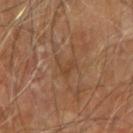patient: male, roughly 70 years of age
image source: ~15 mm tile from a whole-body skin photo
illumination: cross-polarized
anatomic site: the arm
TBP lesion metrics: a mean CIELAB color near L≈42 a*≈19 b*≈31, a lesion–skin lightness drop of about 5, and a normalized border contrast of about 4.5; border irregularity of about 7.5 on a 0–10 scale, internal color variation of about 2 on a 0–10 scale, and peripheral color asymmetry of about 0.5; an automated nevus-likeness rating near 0 out of 100